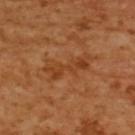Q: Lesion location?
A: the back
Q: What are the patient's age and sex?
A: female, in their mid- to late 50s
Q: What is the imaging modality?
A: ~15 mm crop, total-body skin-cancer survey
Q: Illumination type?
A: cross-polarized illumination
Q: What is the lesion's diameter?
A: ≈6 mm